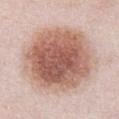{
  "biopsy_status": "not biopsied; imaged during a skin examination"
}